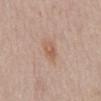automated lesion analysis — a lesion area of about 5 mm², an outline eccentricity of about 0.75 (0 = round, 1 = elongated), and two-axis asymmetry of about 0.2; a border-irregularity rating of about 2/10, a within-lesion color-variation index near 3.5/10, and peripheral color asymmetry of about 1 | illumination — white-light illumination | patient — male, approximately 75 years of age | body site — the abdomen | lesion diameter — ~3 mm (longest diameter) | imaging modality — ~15 mm crop, total-body skin-cancer survey.